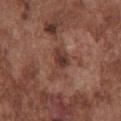Assessment: Recorded during total-body skin imaging; not selected for excision or biopsy. Context: A lesion tile, about 15 mm wide, cut from a 3D total-body photograph. A male patient in their mid- to late 70s. On the chest. An algorithmic analysis of the crop reported a lesion area of about 5.5 mm², an outline eccentricity of about 0.8 (0 = round, 1 = elongated), and a symmetry-axis asymmetry near 0.45. It also reported an average lesion color of about L≈38 a*≈21 b*≈25 (CIELAB), roughly 10 lightness units darker than nearby skin, and a normalized border contrast of about 8. The analysis additionally found border irregularity of about 4.5 on a 0–10 scale and a color-variation rating of about 3.5/10. The analysis additionally found a classifier nevus-likeness of about 5/100. Approximately 3.5 mm at its widest. This is a white-light tile.